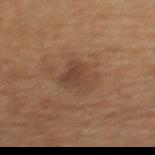Captured during whole-body skin photography for melanoma surveillance; the lesion was not biopsied.
From the upper back.
Cropped from a total-body skin-imaging series; the visible field is about 15 mm.
Automated image analysis of the tile measured a footprint of about 10 mm², an eccentricity of roughly 0.7, and a shape-asymmetry score of about 0.3 (0 = symmetric). The software also gave a lesion-to-skin contrast of about 6.5 (normalized; higher = more distinct). The software also gave a border-irregularity index near 3/10 and internal color variation of about 4.5 on a 0–10 scale. It also reported a detector confidence of about 100 out of 100 that the crop contains a lesion.
The subject is a female in their mid- to late 50s.